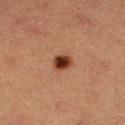Clinical impression:
Part of a total-body skin-imaging series; this lesion was reviewed on a skin check and was not flagged for biopsy.
Acquisition and patient details:
The lesion-visualizer software estimated a lesion area of about 5 mm², an outline eccentricity of about 0.4 (0 = round, 1 = elongated), and a symmetry-axis asymmetry near 0.15. The software also gave a border-irregularity rating of about 1.5/10, a color-variation rating of about 5/10, and peripheral color asymmetry of about 1. On the right lower leg. About 2.5 mm across. A roughly 15 mm field-of-view crop from a total-body skin photograph. The subject is a female aged around 40.